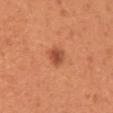{"biopsy_status": "not biopsied; imaged during a skin examination", "lighting": "white-light", "lesion_size": {"long_diameter_mm_approx": 2.5}, "patient": {"sex": "female", "age_approx": 40}, "automated_metrics": {"nevus_likeness_0_100": 95, "lesion_detection_confidence_0_100": 100}, "image": {"source": "total-body photography crop", "field_of_view_mm": 15}, "site": "arm"}